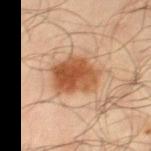The lesion was tiled from a total-body skin photograph and was not biopsied. A roughly 15 mm field-of-view crop from a total-body skin photograph. A male subject in their mid-60s. On the right thigh. Measured at roughly 5 mm in maximum diameter.